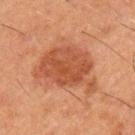subject: male, aged 48–52
imaging modality: ~15 mm crop, total-body skin-cancer survey
body site: the left upper arm
lighting: cross-polarized